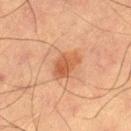| key | value |
|---|---|
| lighting | cross-polarized illumination |
| body site | the right thigh |
| size | about 3.5 mm |
| subject | male, approximately 65 years of age |
| imaging modality | ~15 mm tile from a whole-body skin photo |